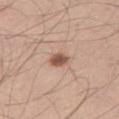Captured during whole-body skin photography for melanoma surveillance; the lesion was not biopsied.
Longest diameter approximately 2.5 mm.
A lesion tile, about 15 mm wide, cut from a 3D total-body photograph.
The patient is a male aged 33 to 37.
Captured under white-light illumination.
The lesion is on the right thigh.
Automated tile analysis of the lesion measured an area of roughly 3.5 mm², an outline eccentricity of about 0.65 (0 = round, 1 = elongated), and a shape-asymmetry score of about 0.2 (0 = symmetric). And it measured a lesion color around L≈54 a*≈21 b*≈28 in CIELAB and a normalized border contrast of about 9.